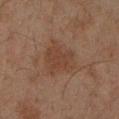Recorded during total-body skin imaging; not selected for excision or biopsy. Automated tile analysis of the lesion measured an area of roughly 12 mm², a shape eccentricity near 0.65, and a symmetry-axis asymmetry near 0.3. The software also gave a mean CIELAB color near L≈32 a*≈16 b*≈23 and a lesion-to-skin contrast of about 5.5 (normalized; higher = more distinct). Located on the left forearm. A lesion tile, about 15 mm wide, cut from a 3D total-body photograph. About 4.5 mm across. A male patient, in their mid- to late 40s. Captured under cross-polarized illumination.